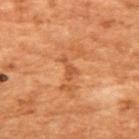<case>
  <biopsy_status>not biopsied; imaged during a skin examination</biopsy_status>
  <image>
    <source>total-body photography crop</source>
    <field_of_view_mm>15</field_of_view_mm>
  </image>
  <lesion_size>
    <long_diameter_mm_approx>2.5</long_diameter_mm_approx>
  </lesion_size>
  <automated_metrics>
    <area_mm2_approx>3.0</area_mm2_approx>
    <eccentricity>0.9</eccentricity>
    <shape_asymmetry>0.45</shape_asymmetry>
    <cielab_L>40</cielab_L>
    <cielab_a>22</cielab_a>
    <cielab_b>32</cielab_b>
    <vs_skin_contrast_norm>5.5</vs_skin_contrast_norm>
    <lesion_detection_confidence_0_100>100</lesion_detection_confidence_0_100>
  </automated_metrics>
  <lighting>cross-polarized</lighting>
  <patient>
    <sex>female</sex>
    <age_approx>80</age_approx>
  </patient>
  <site>front of the torso</site>
</case>